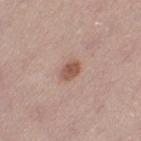Notes:
• notes: catalogued during a skin exam; not biopsied
• location: the left thigh
• illumination: white-light illumination
• patient: female, aged around 30
• diameter: about 2.5 mm
• image: ~15 mm tile from a whole-body skin photo